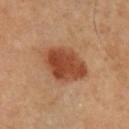Impression:
Part of a total-body skin-imaging series; this lesion was reviewed on a skin check and was not flagged for biopsy.
Context:
Located on the front of the torso. A male subject aged 48–52. Captured under cross-polarized illumination. Automated tile analysis of the lesion measured internal color variation of about 4.5 on a 0–10 scale. A 15 mm close-up extracted from a 3D total-body photography capture. Measured at roughly 5.5 mm in maximum diameter.